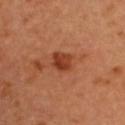Impression: This lesion was catalogued during total-body skin photography and was not selected for biopsy. Background: A region of skin cropped from a whole-body photographic capture, roughly 15 mm wide. Approximately 3 mm at its widest. A female subject, in their mid-30s. Imaged with cross-polarized lighting. Automated image analysis of the tile measured a lesion color around L≈40 a*≈29 b*≈35 in CIELAB and a normalized border contrast of about 8.5. The analysis additionally found border irregularity of about 2 on a 0–10 scale, internal color variation of about 4 on a 0–10 scale, and radial color variation of about 1.5. The software also gave an automated nevus-likeness rating near 80 out of 100 and lesion-presence confidence of about 100/100. On the upper back.